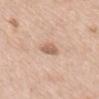Case summary:
- biopsy status: no biopsy performed (imaged during a skin exam)
- automated metrics: a lesion color around L≈61 a*≈20 b*≈30 in CIELAB and a normalized border contrast of about 7; a color-variation rating of about 2/10; a lesion-detection confidence of about 100/100
- tile lighting: white-light
- image: 15 mm crop, total-body photography
- patient: male, about 80 years old
- location: the mid back
- lesion size: ~3 mm (longest diameter)